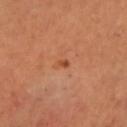Notes:
- follow-up: no biopsy performed (imaged during a skin exam)
- image-analysis metrics: an outline eccentricity of about 0.85 (0 = round, 1 = elongated) and a symmetry-axis asymmetry near 0.35
- image source: 15 mm crop, total-body photography
- patient: male, in their 40s
- anatomic site: the head or neck
- lesion size: ~1.5 mm (longest diameter)